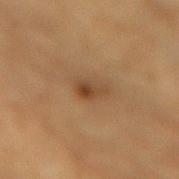Assessment: Part of a total-body skin-imaging series; this lesion was reviewed on a skin check and was not flagged for biopsy. Context: About 2.5 mm across. The lesion is on the mid back. A 15 mm crop from a total-body photograph taken for skin-cancer surveillance. An algorithmic analysis of the crop reported a lesion color around L≈37 a*≈18 b*≈30 in CIELAB, about 8 CIELAB-L* units darker than the surrounding skin, and a lesion-to-skin contrast of about 7.5 (normalized; higher = more distinct). A male patient, aged 83 or over. This is a cross-polarized tile.